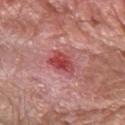Captured during whole-body skin photography for melanoma surveillance; the lesion was not biopsied. A roughly 15 mm field-of-view crop from a total-body skin photograph. A male patient about 75 years old. From the right forearm.